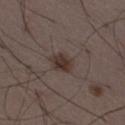The lesion was photographed on a routine skin check and not biopsied; there is no pathology result. Cropped from a whole-body photographic skin survey; the tile spans about 15 mm. Captured under white-light illumination. Located on the right thigh. Longest diameter approximately 3 mm. The lesion-visualizer software estimated an area of roughly 6 mm², an outline eccentricity of about 0.5 (0 = round, 1 = elongated), and two-axis asymmetry of about 0.25. It also reported an average lesion color of about L≈34 a*≈12 b*≈19 (CIELAB) and a normalized border contrast of about 8.5. The patient is a male about 50 years old.